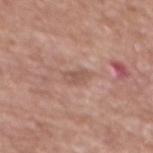biopsy status: total-body-photography surveillance lesion; no biopsy | illumination: white-light | TBP lesion metrics: a lesion color around L≈54 a*≈20 b*≈25 in CIELAB, about 8 CIELAB-L* units darker than the surrounding skin, and a lesion-to-skin contrast of about 5.5 (normalized; higher = more distinct); border irregularity of about 3.5 on a 0–10 scale and a peripheral color-asymmetry measure near 1; an automated nevus-likeness rating near 0 out of 100 and lesion-presence confidence of about 100/100 | image source: total-body-photography crop, ~15 mm field of view | body site: the mid back | size: ≈2.5 mm | subject: female, aged around 75.